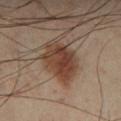biopsy_status: not biopsied; imaged during a skin examination
lesion_size:
  long_diameter_mm_approx: 5.5
image:
  source: total-body photography crop
  field_of_view_mm: 15
site: right lower leg
lighting: cross-polarized
patient:
  sex: male
  age_approx: 40